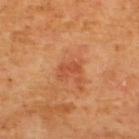<case>
  <biopsy_status>not biopsied; imaged during a skin examination</biopsy_status>
  <site>back</site>
  <lesion_size>
    <long_diameter_mm_approx>2.5</long_diameter_mm_approx>
  </lesion_size>
  <automated_metrics>
    <area_mm2_approx>3.0</area_mm2_approx>
    <eccentricity>0.8</eccentricity>
    <shape_asymmetry>0.4</shape_asymmetry>
    <cielab_L>49</cielab_L>
    <cielab_a>30</cielab_a>
    <cielab_b>38</cielab_b>
    <vs_skin_darker_L>7.0</vs_skin_darker_L>
    <vs_skin_contrast_norm>5.5</vs_skin_contrast_norm>
    <border_irregularity_0_10>5.0</border_irregularity_0_10>
    <color_variation_0_10>0.0</color_variation_0_10>
    <peripheral_color_asymmetry>0.0</peripheral_color_asymmetry>
    <lesion_detection_confidence_0_100>100</lesion_detection_confidence_0_100>
  </automated_metrics>
  <patient>
    <sex>male</sex>
    <age_approx>65</age_approx>
  </patient>
  <lighting>cross-polarized</lighting>
  <image>
    <source>total-body photography crop</source>
    <field_of_view_mm>15</field_of_view_mm>
  </image>
</case>